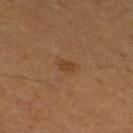Q: Was this lesion biopsied?
A: no biopsy performed (imaged during a skin exam)